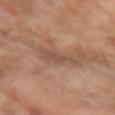The lesion was photographed on a routine skin check and not biopsied; there is no pathology result. A region of skin cropped from a whole-body photographic capture, roughly 15 mm wide. The lesion's longest dimension is about 3.5 mm. Captured under cross-polarized illumination. A female patient in their 70s. On the left forearm.